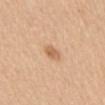The lesion was tiled from a total-body skin photograph and was not biopsied. A female subject, aged 63–67. Automated image analysis of the tile measured an area of roughly 3.5 mm², a shape eccentricity near 0.8, and two-axis asymmetry of about 0.25. And it measured a mean CIELAB color near L≈63 a*≈20 b*≈37, a lesion–skin lightness drop of about 10, and a lesion-to-skin contrast of about 6.5 (normalized; higher = more distinct). It also reported a border-irregularity index near 2/10, a within-lesion color-variation index near 2/10, and radial color variation of about 0.5. And it measured a classifier nevus-likeness of about 70/100. Approximately 2.5 mm at its widest. This image is a 15 mm lesion crop taken from a total-body photograph. The lesion is on the mid back.